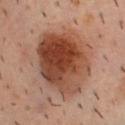Q: How large is the lesion?
A: ≈7.5 mm
Q: What is the imaging modality?
A: 15 mm crop, total-body photography
Q: Patient demographics?
A: male, aged 38 to 42
Q: How was the tile lit?
A: cross-polarized illumination
Q: Where on the body is the lesion?
A: the upper back